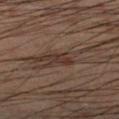This lesion was catalogued during total-body skin photography and was not selected for biopsy.
A male subject, aged around 50.
On the left lower leg.
The recorded lesion diameter is about 3 mm.
A 15 mm close-up tile from a total-body photography series done for melanoma screening.
The tile uses cross-polarized illumination.